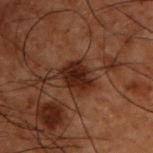{
  "lesion_size": {
    "long_diameter_mm_approx": 3.5
  },
  "patient": {
    "sex": "male",
    "age_approx": 50
  },
  "image": {
    "source": "total-body photography crop",
    "field_of_view_mm": 15
  },
  "automated_metrics": {
    "nevus_likeness_0_100": 65,
    "lesion_detection_confidence_0_100": 100
  },
  "lighting": "cross-polarized",
  "site": "upper back"
}